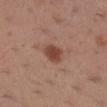follow-up: no biopsy performed (imaged during a skin exam)
automated lesion analysis: a lesion color around L≈44 a*≈23 b*≈28 in CIELAB, roughly 11 lightness units darker than nearby skin, and a lesion-to-skin contrast of about 9 (normalized; higher = more distinct)
imaging modality: ~15 mm crop, total-body skin-cancer survey
lesion diameter: ~3 mm (longest diameter)
body site: the right lower leg
patient: female, aged approximately 30
lighting: white-light illumination